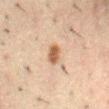diameter=about 2.5 mm | body site=the abdomen | patient=male, approximately 50 years of age | lighting=cross-polarized illumination | image source=total-body-photography crop, ~15 mm field of view.